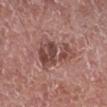automated lesion analysis: an area of roughly 13 mm², an eccentricity of roughly 0.85, and a shape-asymmetry score of about 0.55 (0 = symmetric); border irregularity of about 7.5 on a 0–10 scale, internal color variation of about 5.5 on a 0–10 scale, and peripheral color asymmetry of about 1.5; a classifier nevus-likeness of about 15/100 and a detector confidence of about 100 out of 100 that the crop contains a lesion | diameter: ≈5.5 mm | patient: male, in their mid-70s | image source: 15 mm crop, total-body photography | body site: the right lower leg | tile lighting: white-light.